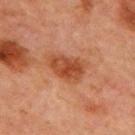Imaged during a routine full-body skin examination; the lesion was not biopsied and no histopathology is available. A region of skin cropped from a whole-body photographic capture, roughly 15 mm wide. This is a cross-polarized tile. The subject is a male about 65 years old. The lesion is on the chest.